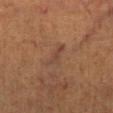The lesion was photographed on a routine skin check and not biopsied; there is no pathology result. A 15 mm crop from a total-body photograph taken for skin-cancer surveillance. Approximately 3.5 mm at its widest. Imaged with cross-polarized lighting. A female subject, in their mid-60s. From the right lower leg.